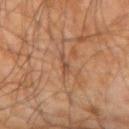This lesion was catalogued during total-body skin photography and was not selected for biopsy. This image is a 15 mm lesion crop taken from a total-body photograph. A male patient, in their mid-60s. The tile uses cross-polarized illumination. The lesion-visualizer software estimated an area of roughly 2 mm², an outline eccentricity of about 0.9 (0 = round, 1 = elongated), and a shape-asymmetry score of about 0.45 (0 = symmetric). The analysis additionally found a mean CIELAB color near L≈41 a*≈18 b*≈28 and a normalized border contrast of about 6. The software also gave an automated nevus-likeness rating near 0 out of 100 and a detector confidence of about 60 out of 100 that the crop contains a lesion. The lesion's longest dimension is about 2.5 mm. On the left forearm.